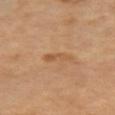biopsy_status: not biopsied; imaged during a skin examination
image:
  source: total-body photography crop
  field_of_view_mm: 15
lesion_size:
  long_diameter_mm_approx: 3.5
site: left upper arm
patient:
  sex: male
  age_approx: 65
lighting: cross-polarized